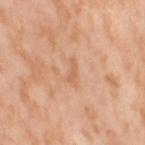<case>
  <patient>
    <sex>female</sex>
    <age_approx>55</age_approx>
  </patient>
  <image>
    <source>total-body photography crop</source>
    <field_of_view_mm>15</field_of_view_mm>
  </image>
  <site>leg</site>
  <lighting>cross-polarized</lighting>
</case>